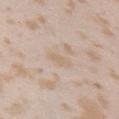The lesion was tiled from a total-body skin photograph and was not biopsied.
A 15 mm close-up tile from a total-body photography series done for melanoma screening.
Imaged with white-light lighting.
A female subject aged approximately 25.
On the right upper arm.
An algorithmic analysis of the crop reported a color-variation rating of about 1.5/10 and radial color variation of about 0.5. It also reported a nevus-likeness score of about 0/100 and a detector confidence of about 95 out of 100 that the crop contains a lesion.